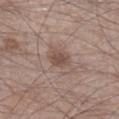follow-up = catalogued during a skin exam; not biopsied | subject = male, aged 43–47 | acquisition = ~15 mm crop, total-body skin-cancer survey | site = the right lower leg | illumination = white-light.